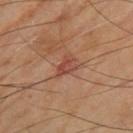| key | value |
|---|---|
| notes | no biopsy performed (imaged during a skin exam) |
| TBP lesion metrics | a lesion color around L≈47 a*≈26 b*≈29 in CIELAB, roughly 9 lightness units darker than nearby skin, and a normalized lesion–skin contrast near 6.5 |
| illumination | cross-polarized |
| body site | the right thigh |
| subject | male, aged approximately 65 |
| image source | 15 mm crop, total-body photography |